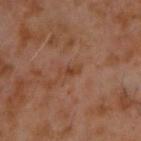{
  "biopsy_status": "not biopsied; imaged during a skin examination",
  "lesion_size": {
    "long_diameter_mm_approx": 2.5
  },
  "site": "upper back",
  "image": {
    "source": "total-body photography crop",
    "field_of_view_mm": 15
  },
  "patient": {
    "sex": "male",
    "age_approx": 60
  },
  "lighting": "cross-polarized"
}